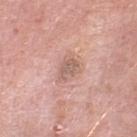Notes:
* notes — no biopsy performed (imaged during a skin exam)
* TBP lesion metrics — an area of roughly 5 mm², a shape eccentricity near 0.7, and a symmetry-axis asymmetry near 0.35; a nevus-likeness score of about 0/100 and lesion-presence confidence of about 100/100
* imaging modality — total-body-photography crop, ~15 mm field of view
* subject — male, aged around 80
* location — the left lower leg